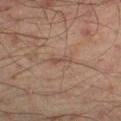Notes:
- workup: total-body-photography surveillance lesion; no biopsy
- size: ~2.5 mm (longest diameter)
- anatomic site: the leg
- patient: male, aged 43 to 47
- image: total-body-photography crop, ~15 mm field of view